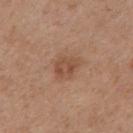Case summary:
– workup — total-body-photography surveillance lesion; no biopsy
– lesion diameter — ≈3 mm
– patient — female, aged 38–42
– site — the upper back
– acquisition — total-body-photography crop, ~15 mm field of view
– tile lighting — white-light illumination
– TBP lesion metrics — a lesion area of about 5.5 mm², a shape eccentricity near 0.6, and two-axis asymmetry of about 0.2; about 8 CIELAB-L* units darker than the surrounding skin and a normalized lesion–skin contrast near 6